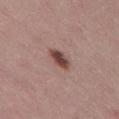| feature | finding |
|---|---|
| biopsy status | no biopsy performed (imaged during a skin exam) |
| image | total-body-photography crop, ~15 mm field of view |
| patient | female, in their mid-40s |
| body site | the right thigh |